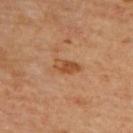Captured during whole-body skin photography for melanoma surveillance; the lesion was not biopsied. The subject is a female about 65 years old. Imaged with cross-polarized lighting. About 3 mm across. A region of skin cropped from a whole-body photographic capture, roughly 15 mm wide. The lesion is located on the upper back.